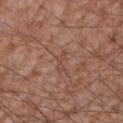Captured during whole-body skin photography for melanoma surveillance; the lesion was not biopsied.
On the right forearm.
A male subject aged 53 to 57.
A 15 mm crop from a total-body photograph taken for skin-cancer surveillance.